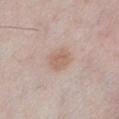biopsy_status: not biopsied; imaged during a skin examination
site: chest
patient:
  sex: male
  age_approx: 25
image:
  source: total-body photography crop
  field_of_view_mm: 15